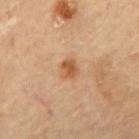The lesion was photographed on a routine skin check and not biopsied; there is no pathology result. The lesion is located on the mid back. Imaged with cross-polarized lighting. A region of skin cropped from a whole-body photographic capture, roughly 15 mm wide. The patient is a male roughly 70 years of age.